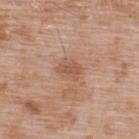Assessment: This lesion was catalogued during total-body skin photography and was not selected for biopsy. Image and clinical context: From the upper back. A male patient about 50 years old. The lesion's longest dimension is about 3 mm. A roughly 15 mm field-of-view crop from a total-body skin photograph.